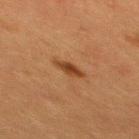The lesion was photographed on a routine skin check and not biopsied; there is no pathology result.
The recorded lesion diameter is about 3 mm.
Automated image analysis of the tile measured a lesion area of about 4 mm². It also reported about 9 CIELAB-L* units darker than the surrounding skin and a lesion-to-skin contrast of about 8.5 (normalized; higher = more distinct). It also reported a nevus-likeness score of about 95/100 and a detector confidence of about 100 out of 100 that the crop contains a lesion.
A male patient approximately 40 years of age.
This image is a 15 mm lesion crop taken from a total-body photograph.
Imaged with cross-polarized lighting.
The lesion is located on the mid back.